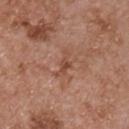Q: Lesion location?
A: the chest
Q: What are the patient's age and sex?
A: male, about 55 years old
Q: What kind of image is this?
A: ~15 mm tile from a whole-body skin photo